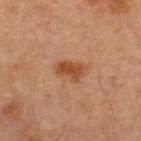The lesion was photographed on a routine skin check and not biopsied; there is no pathology result.
The lesion is on the front of the torso.
Automated image analysis of the tile measured a symmetry-axis asymmetry near 0.25. And it measured a border-irregularity index near 2.5/10, a color-variation rating of about 2.5/10, and peripheral color asymmetry of about 1.
A 15 mm close-up tile from a total-body photography series done for melanoma screening.
Approximately 3.5 mm at its widest.
The tile uses cross-polarized illumination.
A male subject roughly 65 years of age.